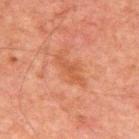follow-up: total-body-photography surveillance lesion; no biopsy
illumination: cross-polarized
patient: male, about 60 years old
image source: 15 mm crop, total-body photography
anatomic site: the back
diameter: ≈4 mm
automated metrics: a lesion area of about 4.5 mm², an eccentricity of roughly 0.9, and a shape-asymmetry score of about 0.35 (0 = symmetric); a border-irregularity rating of about 5/10, internal color variation of about 1 on a 0–10 scale, and a peripheral color-asymmetry measure near 0.5